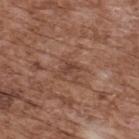Captured during whole-body skin photography for melanoma surveillance; the lesion was not biopsied.
Cropped from a whole-body photographic skin survey; the tile spans about 15 mm.
Automated tile analysis of the lesion measured a lesion color around L≈42 a*≈20 b*≈27 in CIELAB and a lesion–skin lightness drop of about 8.
A male subject, roughly 75 years of age.
About 2.5 mm across.
On the upper back.
The tile uses white-light illumination.